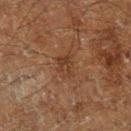follow-up: imaged on a skin check; not biopsied